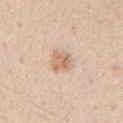{"biopsy_status": "not biopsied; imaged during a skin examination"}